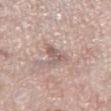Imaged during a routine full-body skin examination; the lesion was not biopsied and no histopathology is available.
About 3.5 mm across.
From the left thigh.
Cropped from a whole-body photographic skin survey; the tile spans about 15 mm.
The subject is a male roughly 80 years of age.
Captured under white-light illumination.